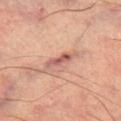No biopsy was performed on this lesion — it was imaged during a full skin examination and was not determined to be concerning.
A male subject, aged approximately 65.
The lesion is on the leg.
Approximately 3.5 mm at its widest.
The total-body-photography lesion software estimated an average lesion color of about L≈56 a*≈26 b*≈26 (CIELAB) and a lesion-to-skin contrast of about 8.5 (normalized; higher = more distinct). The software also gave border irregularity of about 4 on a 0–10 scale, internal color variation of about 0 on a 0–10 scale, and peripheral color asymmetry of about 0. The analysis additionally found a nevus-likeness score of about 0/100 and a detector confidence of about 65 out of 100 that the crop contains a lesion.
Imaged with cross-polarized lighting.
This image is a 15 mm lesion crop taken from a total-body photograph.